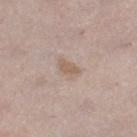No biopsy was performed on this lesion — it was imaged during a full skin examination and was not determined to be concerning.
On the right lower leg.
A lesion tile, about 15 mm wide, cut from a 3D total-body photograph.
A female subject aged approximately 40.